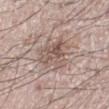Assessment:
The lesion was photographed on a routine skin check and not biopsied; there is no pathology result.
Clinical summary:
The subject is a male about 70 years old. A lesion tile, about 15 mm wide, cut from a 3D total-body photograph. On the right leg.